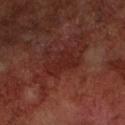notes = no biopsy performed (imaged during a skin exam) | subject = male, aged 63–67 | diameter = ~4 mm (longest diameter) | imaging modality = total-body-photography crop, ~15 mm field of view | body site = the left forearm.